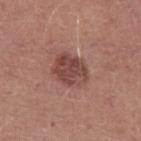{
  "biopsy_status": "not biopsied; imaged during a skin examination",
  "lesion_size": {
    "long_diameter_mm_approx": 4.0
  },
  "patient": {
    "sex": "male",
    "age_approx": 25
  },
  "automated_metrics": {
    "border_irregularity_0_10": 2.5,
    "color_variation_0_10": 4.5
  },
  "lighting": "white-light",
  "site": "left upper arm",
  "image": {
    "source": "total-body photography crop",
    "field_of_view_mm": 15
  }
}